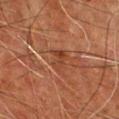{"biopsy_status": "not biopsied; imaged during a skin examination", "site": "chest", "lesion_size": {"long_diameter_mm_approx": 2.5}, "image": {"source": "total-body photography crop", "field_of_view_mm": 15}, "lighting": "cross-polarized", "patient": {"sex": "male", "age_approx": 65}}